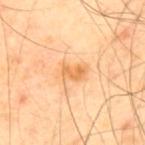No biopsy was performed on this lesion — it was imaged during a full skin examination and was not determined to be concerning.
The recorded lesion diameter is about 3.5 mm.
The lesion is on the upper back.
The subject is a male in their 40s.
Imaged with cross-polarized lighting.
Cropped from a total-body skin-imaging series; the visible field is about 15 mm.